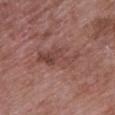Captured during whole-body skin photography for melanoma surveillance; the lesion was not biopsied.
A female subject aged 68–72.
Automated tile analysis of the lesion measured an area of roughly 11 mm², a shape eccentricity near 0.9, and a shape-asymmetry score of about 0.4 (0 = symmetric).
The lesion is on the right upper arm.
Longest diameter approximately 6 mm.
A 15 mm crop from a total-body photograph taken for skin-cancer surveillance.
This is a white-light tile.